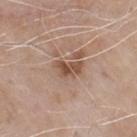{
  "biopsy_status": "not biopsied; imaged during a skin examination",
  "patient": {
    "sex": "male",
    "age_approx": 75
  },
  "site": "chest",
  "lesion_size": {
    "long_diameter_mm_approx": 3.0
  },
  "image": {
    "source": "total-body photography crop",
    "field_of_view_mm": 15
  },
  "lighting": "white-light",
  "automated_metrics": {
    "area_mm2_approx": 5.0,
    "eccentricity": 0.8,
    "shape_asymmetry": 0.45,
    "cielab_L": 51,
    "cielab_a": 19,
    "cielab_b": 29,
    "nevus_likeness_0_100": 15,
    "lesion_detection_confidence_0_100": 100
  }
}